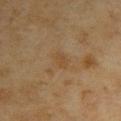Findings:
• biopsy status — imaged on a skin check; not biopsied
• body site — the right upper arm
• patient — female, aged 58 to 62
• tile lighting — cross-polarized illumination
• image — ~15 mm tile from a whole-body skin photo
• TBP lesion metrics — an eccentricity of roughly 0.8 and a symmetry-axis asymmetry near 0.25; a lesion–skin lightness drop of about 5 and a normalized border contrast of about 5; a border-irregularity index near 2.5/10, internal color variation of about 1 on a 0–10 scale, and peripheral color asymmetry of about 0.5
• diameter — ~2.5 mm (longest diameter)